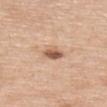The lesion was photographed on a routine skin check and not biopsied; there is no pathology result.
A 15 mm close-up extracted from a 3D total-body photography capture.
The lesion is on the upper back.
A female patient, in their mid-60s.
The lesion-visualizer software estimated an eccentricity of roughly 0.8. It also reported a lesion color around L≈58 a*≈20 b*≈32 in CIELAB, about 14 CIELAB-L* units darker than the surrounding skin, and a normalized border contrast of about 8.5. The analysis additionally found an automated nevus-likeness rating near 85 out of 100 and a detector confidence of about 100 out of 100 that the crop contains a lesion.